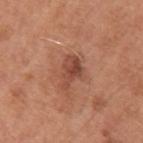Q: Was a biopsy performed?
A: total-body-photography surveillance lesion; no biopsy
Q: What did automated image analysis measure?
A: a mean CIELAB color near L≈47 a*≈25 b*≈30, a lesion–skin lightness drop of about 10, and a lesion-to-skin contrast of about 7.5 (normalized; higher = more distinct); a border-irregularity index near 4/10, internal color variation of about 5 on a 0–10 scale, and radial color variation of about 2; an automated nevus-likeness rating near 20 out of 100 and lesion-presence confidence of about 100/100
Q: Where on the body is the lesion?
A: the left upper arm
Q: Who is the patient?
A: male, aged 63–67
Q: What lighting was used for the tile?
A: white-light
Q: Lesion size?
A: about 4 mm
Q: What is the imaging modality?
A: ~15 mm tile from a whole-body skin photo